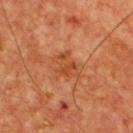No biopsy was performed on this lesion — it was imaged during a full skin examination and was not determined to be concerning.
A 15 mm close-up extracted from a 3D total-body photography capture.
About 3.5 mm across.
The lesion-visualizer software estimated an area of roughly 8 mm². The software also gave a mean CIELAB color near L≈45 a*≈27 b*≈37, roughly 7 lightness units darker than nearby skin, and a lesion-to-skin contrast of about 6 (normalized; higher = more distinct). The software also gave a border-irregularity rating of about 4.5/10, a color-variation rating of about 3.5/10, and radial color variation of about 1. The software also gave a nevus-likeness score of about 0/100 and a lesion-detection confidence of about 100/100.
The patient is a male approximately 55 years of age.
Imaged with cross-polarized lighting.
From the chest.